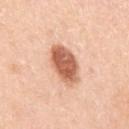  biopsy_status: not biopsied; imaged during a skin examination
  patient:
    sex: female
    age_approx: 45
  lesion_size:
    long_diameter_mm_approx: 4.5
  site: arm
  automated_metrics:
    area_mm2_approx: 12.0
    shape_asymmetry: 0.15
    cielab_L: 61
    cielab_a: 26
    cielab_b: 34
    vs_skin_darker_L: 18.0
    vs_skin_contrast_norm: 10.5
    border_irregularity_0_10: 1.5
    color_variation_0_10: 4.5
    peripheral_color_asymmetry: 1.5
    lesion_detection_confidence_0_100: 100
  lighting: white-light
  image:
    source: total-body photography crop
    field_of_view_mm: 15